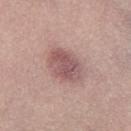Impression:
Part of a total-body skin-imaging series; this lesion was reviewed on a skin check and was not flagged for biopsy.
Clinical summary:
On the left thigh. A lesion tile, about 15 mm wide, cut from a 3D total-body photograph. Measured at roughly 4.5 mm in maximum diameter. Automated image analysis of the tile measured a shape eccentricity near 0.6 and a symmetry-axis asymmetry near 0.15. And it measured a mean CIELAB color near L≈54 a*≈21 b*≈20 and a lesion-to-skin contrast of about 7.5 (normalized; higher = more distinct). The software also gave a border-irregularity rating of about 1.5/10, a color-variation rating of about 4/10, and a peripheral color-asymmetry measure near 1.5. And it measured a classifier nevus-likeness of about 35/100 and a lesion-detection confidence of about 100/100. Captured under white-light illumination. A female subject, aged approximately 55.